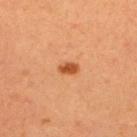The lesion was tiled from a total-body skin photograph and was not biopsied.
Cropped from a whole-body photographic skin survey; the tile spans about 15 mm.
A male subject aged approximately 35.
From the upper back.
Automated tile analysis of the lesion measured an eccentricity of roughly 0.85 and a symmetry-axis asymmetry near 0.15.